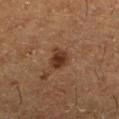Imaged during a routine full-body skin examination; the lesion was not biopsied and no histopathology is available.
The lesion's longest dimension is about 3.5 mm.
The lesion is on the left lower leg.
A 15 mm crop from a total-body photograph taken for skin-cancer surveillance.
The patient is a female in their 50s.